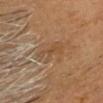Imaged during a routine full-body skin examination; the lesion was not biopsied and no histopathology is available. On the head or neck. A roughly 15 mm field-of-view crop from a total-body skin photograph. Captured under cross-polarized illumination. A male patient in their mid- to late 60s. Longest diameter approximately 3.5 mm. An algorithmic analysis of the crop reported an area of roughly 6 mm², an eccentricity of roughly 0.65, and a shape-asymmetry score of about 0.35 (0 = symmetric). It also reported an average lesion color of about L≈42 a*≈16 b*≈30 (CIELAB), roughly 5 lightness units darker than nearby skin, and a normalized border contrast of about 4.5.